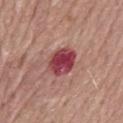Part of a total-body skin-imaging series; this lesion was reviewed on a skin check and was not flagged for biopsy. The lesion is on the mid back. Cropped from a whole-body photographic skin survey; the tile spans about 15 mm. Imaged with white-light lighting. Approximately 4 mm at its widest. An algorithmic analysis of the crop reported an area of roughly 9.5 mm², a shape eccentricity near 0.55, and two-axis asymmetry of about 0.15. And it measured an average lesion color of about L≈43 a*≈34 b*≈20 (CIELAB), roughly 16 lightness units darker than nearby skin, and a normalized lesion–skin contrast near 12. And it measured an automated nevus-likeness rating near 0 out of 100 and lesion-presence confidence of about 100/100. The subject is a male aged approximately 75.